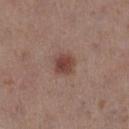biopsy status = total-body-photography surveillance lesion; no biopsy | subject = female, aged 38 to 42 | size = ≈2.5 mm | imaging modality = ~15 mm tile from a whole-body skin photo | site = the leg.